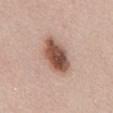This lesion was catalogued during total-body skin photography and was not selected for biopsy. Located on the abdomen. A female subject aged 28–32. A 15 mm crop from a total-body photograph taken for skin-cancer surveillance. The recorded lesion diameter is about 5 mm.